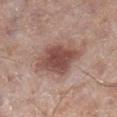Recorded during total-body skin imaging; not selected for excision or biopsy.